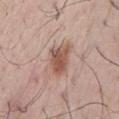{
  "biopsy_status": "not biopsied; imaged during a skin examination",
  "automated_metrics": {
    "area_mm2_approx": 8.5,
    "eccentricity": 0.75,
    "shape_asymmetry": 0.25,
    "cielab_L": 54,
    "cielab_a": 20,
    "cielab_b": 27,
    "vs_skin_darker_L": 12.0,
    "vs_skin_contrast_norm": 8.5,
    "nevus_likeness_0_100": 95,
    "lesion_detection_confidence_0_100": 100
  },
  "patient": {
    "sex": "male",
    "age_approx": 60
  },
  "site": "chest",
  "lighting": "white-light",
  "image": {
    "source": "total-body photography crop",
    "field_of_view_mm": 15
  },
  "lesion_size": {
    "long_diameter_mm_approx": 4.0
  }
}